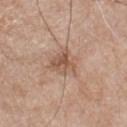notes — no biopsy performed (imaged during a skin exam); subject — male, in their mid- to late 60s; diameter — ~3.5 mm (longest diameter); site — the chest; image source — total-body-photography crop, ~15 mm field of view.